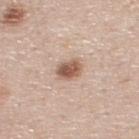biopsy status: total-body-photography surveillance lesion; no biopsy | size: ~3 mm (longest diameter) | tile lighting: white-light | automated lesion analysis: a footprint of about 5.5 mm², an eccentricity of roughly 0.7, and a symmetry-axis asymmetry near 0.15; a lesion color around L≈57 a*≈19 b*≈28 in CIELAB and about 14 CIELAB-L* units darker than the surrounding skin; a within-lesion color-variation index near 4.5/10 and peripheral color asymmetry of about 1.5 | site: the back | subject: male, approximately 45 years of age | image source: ~15 mm tile from a whole-body skin photo.